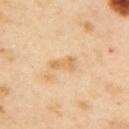{
  "biopsy_status": "not biopsied; imaged during a skin examination",
  "image": {
    "source": "total-body photography crop",
    "field_of_view_mm": 15
  },
  "patient": {
    "sex": "female",
    "age_approx": 40
  },
  "lesion_size": {
    "long_diameter_mm_approx": 3.5
  },
  "automated_metrics": {
    "area_mm2_approx": 4.0,
    "eccentricity": 0.9,
    "shape_asymmetry": 0.35,
    "cielab_L": 71,
    "cielab_a": 18,
    "cielab_b": 42,
    "vs_skin_darker_L": 8.0,
    "vs_skin_contrast_norm": 6.0,
    "color_variation_0_10": 1.0
  },
  "site": "left arm",
  "lighting": "cross-polarized"
}